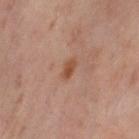| field | value |
|---|---|
| anatomic site | the right thigh |
| subject | female, aged around 50 |
| illumination | cross-polarized |
| lesion diameter | ~2.5 mm (longest diameter) |
| image | ~15 mm tile from a whole-body skin photo |